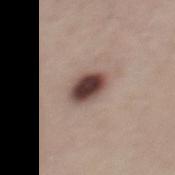biopsy_status: not biopsied; imaged during a skin examination
site: mid back
lighting: white-light
patient:
  sex: male
  age_approx: 35
image:
  source: total-body photography crop
  field_of_view_mm: 15
automated_metrics:
  border_irregularity_0_10: 1.5
  peripheral_color_asymmetry: 1.5
lesion_size:
  long_diameter_mm_approx: 4.0